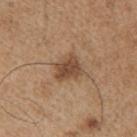Notes:
• notes — no biopsy performed (imaged during a skin exam)
• imaging modality — total-body-photography crop, ~15 mm field of view
• patient — male, about 65 years old
• TBP lesion metrics — about 11 CIELAB-L* units darker than the surrounding skin and a normalized lesion–skin contrast near 8
• tile lighting — white-light illumination
• diameter — ~3.5 mm (longest diameter)
• body site — the arm